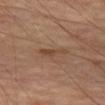Notes:
• workup — catalogued during a skin exam; not biopsied
• subject — male, aged around 70
• body site — the leg
• imaging modality — 15 mm crop, total-body photography
• lesion diameter — about 3.5 mm
• tile lighting — cross-polarized